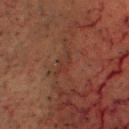Assessment: This lesion was catalogued during total-body skin photography and was not selected for biopsy. Acquisition and patient details: This image is a 15 mm lesion crop taken from a total-body photograph. The lesion is located on the head or neck. A male patient, approximately 60 years of age.